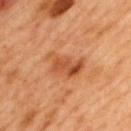Recorded during total-body skin imaging; not selected for excision or biopsy. On the mid back. A 15 mm close-up extracted from a 3D total-body photography capture. Approximately 5 mm at its widest. Automated image analysis of the tile measured an area of roughly 10 mm² and a symmetry-axis asymmetry near 0.3. The analysis additionally found roughly 10 lightness units darker than nearby skin. The analysis additionally found border irregularity of about 4 on a 0–10 scale, a within-lesion color-variation index near 6.5/10, and peripheral color asymmetry of about 2.5. And it measured an automated nevus-likeness rating near 10 out of 100 and lesion-presence confidence of about 100/100. Imaged with cross-polarized lighting. The subject is a male aged approximately 50.